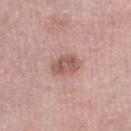The lesion was tiled from a total-body skin photograph and was not biopsied.
A female patient about 70 years old.
Captured under white-light illumination.
The recorded lesion diameter is about 3.5 mm.
On the left lower leg.
A 15 mm close-up extracted from a 3D total-body photography capture.